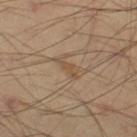This lesion was catalogued during total-body skin photography and was not selected for biopsy. On the left thigh. A male subject aged 38–42. The recorded lesion diameter is about 3 mm. Cropped from a total-body skin-imaging series; the visible field is about 15 mm.